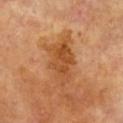Recorded during total-body skin imaging; not selected for excision or biopsy. Cropped from a whole-body photographic skin survey; the tile spans about 15 mm. On the upper back. A female subject, in their mid- to late 70s. Imaged with cross-polarized lighting.